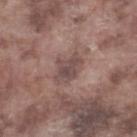Clinical impression: Imaged during a routine full-body skin examination; the lesion was not biopsied and no histopathology is available. Context: Measured at roughly 3.5 mm in maximum diameter. On the right lower leg. A 15 mm crop from a total-body photograph taken for skin-cancer surveillance. A male subject aged around 75.